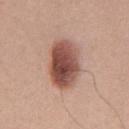<case>
  <biopsy_status>not biopsied; imaged during a skin examination</biopsy_status>
  <image>
    <source>total-body photography crop</source>
    <field_of_view_mm>15</field_of_view_mm>
  </image>
  <lighting>white-light</lighting>
  <site>abdomen</site>
  <patient>
    <sex>male</sex>
    <age_approx>25</age_approx>
  </patient>
  <lesion_size>
    <long_diameter_mm_approx>6.0</long_diameter_mm_approx>
  </lesion_size>
</case>